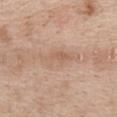follow-up — catalogued during a skin exam; not biopsied
image — ~15 mm tile from a whole-body skin photo
body site — the chest
patient — female, in their 40s